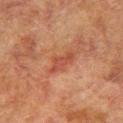The lesion is located on the arm. A 15 mm crop from a total-body photograph taken for skin-cancer surveillance. A male subject, approximately 75 years of age.